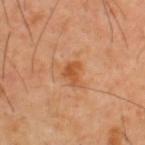This lesion was catalogued during total-body skin photography and was not selected for biopsy.
The patient is a male in their 40s.
Cropped from a total-body skin-imaging series; the visible field is about 15 mm.
Imaged with cross-polarized lighting.
From the upper back.
An algorithmic analysis of the crop reported a lesion area of about 3.5 mm² and a shape eccentricity near 0.7. It also reported border irregularity of about 4.5 on a 0–10 scale, a within-lesion color-variation index near 1.5/10, and peripheral color asymmetry of about 0.5.